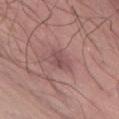<tbp_lesion>
<biopsy_status>not biopsied; imaged during a skin examination</biopsy_status>
<site>abdomen</site>
<patient>
  <sex>male</sex>
  <age_approx>55</age_approx>
</patient>
<image>
  <source>total-body photography crop</source>
  <field_of_view_mm>15</field_of_view_mm>
</image>
</tbp_lesion>